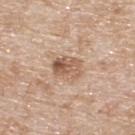Assessment: Imaged during a routine full-body skin examination; the lesion was not biopsied and no histopathology is available. Background: A lesion tile, about 15 mm wide, cut from a 3D total-body photograph. This is a white-light tile. A male patient aged 78 to 82. The lesion is located on the upper back. Approximately 4 mm at its widest. The lesion-visualizer software estimated a footprint of about 8 mm², an outline eccentricity of about 0.75 (0 = round, 1 = elongated), and two-axis asymmetry of about 0.2. The software also gave a mean CIELAB color near L≈58 a*≈18 b*≈30 and a lesion–skin lightness drop of about 11.